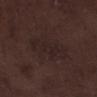follow-up = no biopsy performed (imaged during a skin exam); site = the left lower leg; subject = male, in their 70s; imaging modality = ~15 mm crop, total-body skin-cancer survey.